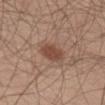| feature | finding |
|---|---|
| biopsy status | catalogued during a skin exam; not biopsied |
| patient | male, in their 60s |
| image | ~15 mm tile from a whole-body skin photo |
| anatomic site | the left thigh |